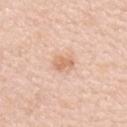The lesion was tiled from a total-body skin photograph and was not biopsied. Measured at roughly 2.5 mm in maximum diameter. The patient is a female roughly 45 years of age. The tile uses white-light illumination. From the right upper arm. A roughly 15 mm field-of-view crop from a total-body skin photograph.